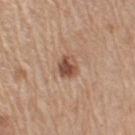Part of a total-body skin-imaging series; this lesion was reviewed on a skin check and was not flagged for biopsy. The patient is a male aged approximately 65. A region of skin cropped from a whole-body photographic capture, roughly 15 mm wide. About 3 mm across. Automated tile analysis of the lesion measured radial color variation of about 1.5. It also reported a detector confidence of about 100 out of 100 that the crop contains a lesion. From the right upper arm. The tile uses white-light illumination.